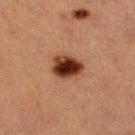follow-up: no biopsy performed (imaged during a skin exam)
image source: total-body-photography crop, ~15 mm field of view
TBP lesion metrics: a footprint of about 8.5 mm², an eccentricity of roughly 0.65, and a shape-asymmetry score of about 0.15 (0 = symmetric); a lesion color around L≈30 a*≈22 b*≈26 in CIELAB, roughly 17 lightness units darker than nearby skin, and a lesion-to-skin contrast of about 15 (normalized; higher = more distinct); border irregularity of about 1.5 on a 0–10 scale, a color-variation rating of about 7.5/10, and radial color variation of about 2; an automated nevus-likeness rating near 100 out of 100 and lesion-presence confidence of about 100/100
tile lighting: cross-polarized
lesion size: about 3.5 mm
patient: female, aged 38 to 42
site: the right lower leg